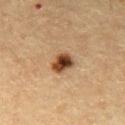Notes:
– image · 15 mm crop, total-body photography
– lesion diameter · ≈3 mm
– patient · male, aged around 60
– automated lesion analysis · a footprint of about 5.5 mm² and a shape-asymmetry score of about 0.2 (0 = symmetric); an average lesion color of about L≈39 a*≈19 b*≈31 (CIELAB), a lesion–skin lightness drop of about 17, and a lesion-to-skin contrast of about 13 (normalized; higher = more distinct)
– lighting · cross-polarized